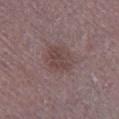This lesion was catalogued during total-body skin photography and was not selected for biopsy. A 15 mm crop from a total-body photograph taken for skin-cancer surveillance. A male subject aged approximately 70. From the leg. This is a white-light tile. The lesion's longest dimension is about 3.5 mm.